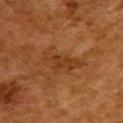follow-up = no biopsy performed (imaged during a skin exam) | patient = female, about 50 years old | location = the upper back | image source = total-body-photography crop, ~15 mm field of view.